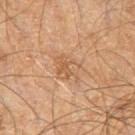workup: catalogued during a skin exam; not biopsied | patient: male, aged 58 to 62 | body site: the right leg | lighting: cross-polarized illumination | diameter: ~3.5 mm (longest diameter) | image: 15 mm crop, total-body photography.